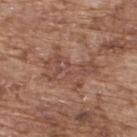Q: Was this lesion biopsied?
A: imaged on a skin check; not biopsied
Q: How was the tile lit?
A: white-light illumination
Q: Who is the patient?
A: male, aged 73–77
Q: What is the imaging modality?
A: total-body-photography crop, ~15 mm field of view
Q: Automated lesion metrics?
A: a shape eccentricity near 0.9 and a shape-asymmetry score of about 0.6 (0 = symmetric); an average lesion color of about L≈47 a*≈21 b*≈26 (CIELAB); border irregularity of about 8.5 on a 0–10 scale, a color-variation rating of about 3.5/10, and a peripheral color-asymmetry measure near 1
Q: Where on the body is the lesion?
A: the upper back
Q: How large is the lesion?
A: ≈6 mm